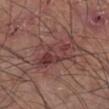Assessment: Captured during whole-body skin photography for melanoma surveillance; the lesion was not biopsied. Context: A close-up tile cropped from a whole-body skin photograph, about 15 mm across. Automated tile analysis of the lesion measured a shape eccentricity near 0.9 and a symmetry-axis asymmetry near 0.3. It also reported a lesion color around L≈39 a*≈23 b*≈20 in CIELAB and about 9 CIELAB-L* units darker than the surrounding skin. The analysis additionally found border irregularity of about 4.5 on a 0–10 scale, a color-variation rating of about 6/10, and peripheral color asymmetry of about 2. It also reported lesion-presence confidence of about 85/100. The lesion is located on the left lower leg. A male patient in their mid-40s.